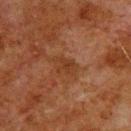| field | value |
|---|---|
| biopsy status | catalogued during a skin exam; not biopsied |
| anatomic site | the back |
| image source | 15 mm crop, total-body photography |
| subject | male, approximately 80 years of age |